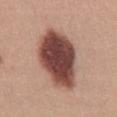Imaged during a routine full-body skin examination; the lesion was not biopsied and no histopathology is available.
A 15 mm close-up tile from a total-body photography series done for melanoma screening.
The lesion is on the mid back.
A female subject, about 35 years old.
The tile uses white-light illumination.
The lesion-visualizer software estimated an area of roughly 30 mm², an outline eccentricity of about 0.85 (0 = round, 1 = elongated), and a shape-asymmetry score of about 0.2 (0 = symmetric). The software also gave an average lesion color of about L≈45 a*≈23 b*≈23 (CIELAB), about 21 CIELAB-L* units darker than the surrounding skin, and a normalized border contrast of about 14.5. And it measured a nevus-likeness score of about 90/100 and a lesion-detection confidence of about 100/100.